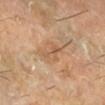This lesion was catalogued during total-body skin photography and was not selected for biopsy. Measured at roughly 3.5 mm in maximum diameter. A 15 mm close-up extracted from a 3D total-body photography capture. A male patient, aged approximately 60. Captured under cross-polarized illumination. The lesion is on the leg.